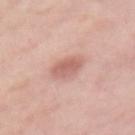The lesion was photographed on a routine skin check and not biopsied; there is no pathology result. A female patient aged 63–67. The lesion is on the mid back. Cropped from a whole-body photographic skin survey; the tile spans about 15 mm.